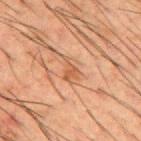The lesion was tiled from a total-body skin photograph and was not biopsied. Cropped from a whole-body photographic skin survey; the tile spans about 15 mm. Measured at roughly 2.5 mm in maximum diameter. A male subject about 50 years old. From the upper back. This is a cross-polarized tile.